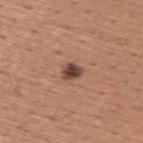Impression:
This lesion was catalogued during total-body skin photography and was not selected for biopsy.
Clinical summary:
A 15 mm close-up extracted from a 3D total-body photography capture. This is a white-light tile. A male patient aged around 45. Measured at roughly 2.5 mm in maximum diameter. From the upper back.